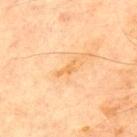Q: Is there a histopathology result?
A: imaged on a skin check; not biopsied
Q: How large is the lesion?
A: ~3 mm (longest diameter)
Q: How was the tile lit?
A: cross-polarized
Q: Who is the patient?
A: male, roughly 70 years of age
Q: How was this image acquired?
A: ~15 mm tile from a whole-body skin photo
Q: What did automated image analysis measure?
A: a border-irregularity index near 6/10, a color-variation rating of about 0/10, and a peripheral color-asymmetry measure near 0
Q: Where on the body is the lesion?
A: the front of the torso